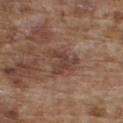Captured during whole-body skin photography for melanoma surveillance; the lesion was not biopsied.